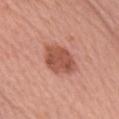{"lesion_size": {"long_diameter_mm_approx": 5.0}, "image": {"source": "total-body photography crop", "field_of_view_mm": 15}, "patient": {"sex": "female", "age_approx": 60}, "site": "front of the torso"}